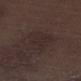- biopsy status — no biopsy performed (imaged during a skin exam)
- acquisition — ~15 mm crop, total-body skin-cancer survey
- patient — male, aged 68–72
- tile lighting — white-light illumination
- lesion diameter — ≈5 mm
- site — the leg
- automated lesion analysis — an eccentricity of roughly 0.8 and two-axis asymmetry of about 0.3; lesion-presence confidence of about 95/100